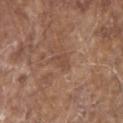Acquisition and patient details:
Automated image analysis of the tile measured a border-irregularity rating of about 3.5/10 and a color-variation rating of about 1.5/10. The patient is a male roughly 80 years of age. About 3 mm across. Cropped from a whole-body photographic skin survey; the tile spans about 15 mm. From the head or neck.